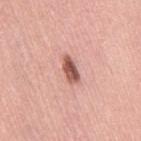biopsy_status: not biopsied; imaged during a skin examination
patient:
  sex: female
  age_approx: 65
lighting: white-light
lesion_size:
  long_diameter_mm_approx: 3.5
image:
  source: total-body photography crop
  field_of_view_mm: 15
site: right thigh